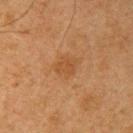Findings:
* lesion size · ~3 mm (longest diameter)
* body site · the right upper arm
* lighting · cross-polarized illumination
* subject · male, aged around 65
* acquisition · 15 mm crop, total-body photography